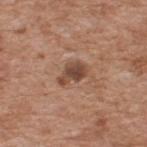{
  "biopsy_status": "not biopsied; imaged during a skin examination",
  "image": {
    "source": "total-body photography crop",
    "field_of_view_mm": 15
  },
  "lighting": "white-light",
  "patient": {
    "sex": "male",
    "age_approx": 60
  },
  "site": "upper back",
  "automated_metrics": {
    "area_mm2_approx": 5.5,
    "eccentricity": 0.8,
    "shape_asymmetry": 0.35,
    "nevus_likeness_0_100": 50
  },
  "lesion_size": {
    "long_diameter_mm_approx": 3.5
  }
}